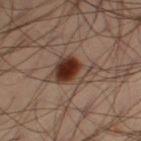- workup: total-body-photography surveillance lesion; no biopsy
- acquisition: ~15 mm tile from a whole-body skin photo
- subject: male, approximately 40 years of age
- lighting: cross-polarized
- location: the left thigh